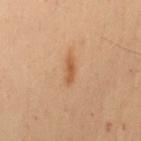Clinical impression: Captured during whole-body skin photography for melanoma surveillance; the lesion was not biopsied. Context: About 3.5 mm across. A female patient, about 50 years old. The lesion-visualizer software estimated a lesion area of about 3.5 mm² and a shape-asymmetry score of about 0.25 (0 = symmetric). The analysis additionally found a mean CIELAB color near L≈48 a*≈20 b*≈33, roughly 8 lightness units darker than nearby skin, and a normalized border contrast of about 7. The software also gave border irregularity of about 3 on a 0–10 scale and radial color variation of about 0. It also reported a classifier nevus-likeness of about 95/100 and a lesion-detection confidence of about 100/100. The lesion is located on the mid back. Cropped from a whole-body photographic skin survey; the tile spans about 15 mm. Captured under cross-polarized illumination.